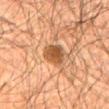Part of a total-body skin-imaging series; this lesion was reviewed on a skin check and was not flagged for biopsy. A male patient aged 58 to 62. A region of skin cropped from a whole-body photographic capture, roughly 15 mm wide. The total-body-photography lesion software estimated a lesion area of about 7 mm², a shape eccentricity near 0.65, and two-axis asymmetry of about 0.2. And it measured a nevus-likeness score of about 75/100. From the mid back. Captured under cross-polarized illumination.